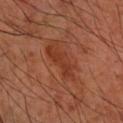The subject is a male roughly 60 years of age. The recorded lesion diameter is about 5 mm. The lesion is on the right forearm. Imaged with cross-polarized lighting. A roughly 15 mm field-of-view crop from a total-body skin photograph.